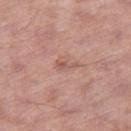biopsy_status: not biopsied; imaged during a skin examination
automated_metrics:
  area_mm2_approx: 2.0
  eccentricity: 0.95
  cielab_L: 56
  cielab_a: 23
  cielab_b: 26
  vs_skin_contrast_norm: 5.5
  color_variation_0_10: 0.0
  peripheral_color_asymmetry: 0.0
lighting: white-light
site: right thigh
patient:
  sex: female
  age_approx: 60
image:
  source: total-body photography crop
  field_of_view_mm: 15
lesion_size:
  long_diameter_mm_approx: 2.5